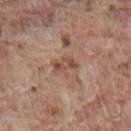Assessment:
Captured during whole-body skin photography for melanoma surveillance; the lesion was not biopsied.
Background:
From the lower back. The patient is a male roughly 80 years of age. A 15 mm close-up extracted from a 3D total-body photography capture.